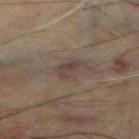  biopsy_status: not biopsied; imaged during a skin examination
  patient:
    sex: male
    age_approx: 60
  image:
    source: total-body photography crop
    field_of_view_mm: 15
  lesion_size:
    long_diameter_mm_approx: 3.0
  lighting: cross-polarized
  site: left thigh